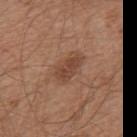Assessment: Captured during whole-body skin photography for melanoma surveillance; the lesion was not biopsied. Background: The lesion is located on the back. The patient is a male aged approximately 65. A region of skin cropped from a whole-body photographic capture, roughly 15 mm wide. Imaged with white-light lighting. The total-body-photography lesion software estimated a lesion color around L≈44 a*≈21 b*≈29 in CIELAB, a lesion–skin lightness drop of about 9, and a lesion-to-skin contrast of about 7 (normalized; higher = more distinct). The analysis additionally found border irregularity of about 2.5 on a 0–10 scale, internal color variation of about 2 on a 0–10 scale, and a peripheral color-asymmetry measure near 0.5. Approximately 3.5 mm at its widest.